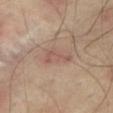Notes:
• workup — catalogued during a skin exam; not biopsied
• site — the right thigh
• acquisition — total-body-photography crop, ~15 mm field of view
• diameter — ≈4 mm